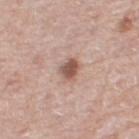Clinical impression:
The lesion was tiled from a total-body skin photograph and was not biopsied.
Context:
The lesion's longest dimension is about 2.5 mm. From the right thigh. A 15 mm crop from a total-body photograph taken for skin-cancer surveillance. The subject is a female roughly 65 years of age.